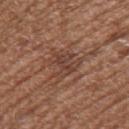Q: Is there a histopathology result?
A: total-body-photography surveillance lesion; no biopsy
Q: Lesion size?
A: ~4 mm (longest diameter)
Q: What did automated image analysis measure?
A: a footprint of about 8.5 mm² and two-axis asymmetry of about 0.45; a mean CIELAB color near L≈42 a*≈20 b*≈26, roughly 7 lightness units darker than nearby skin, and a lesion-to-skin contrast of about 6 (normalized; higher = more distinct); a classifier nevus-likeness of about 5/100 and a lesion-detection confidence of about 70/100
Q: What are the patient's age and sex?
A: female, approximately 80 years of age
Q: Lesion location?
A: the left upper arm
Q: How was this image acquired?
A: 15 mm crop, total-body photography